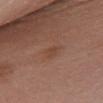Q: Was this lesion biopsied?
A: imaged on a skin check; not biopsied
Q: Lesion location?
A: the chest
Q: How large is the lesion?
A: ≈2.5 mm
Q: What is the imaging modality?
A: ~15 mm crop, total-body skin-cancer survey
Q: What did automated image analysis measure?
A: border irregularity of about 2 on a 0–10 scale and internal color variation of about 2 on a 0–10 scale; a nevus-likeness score of about 0/100
Q: What are the patient's age and sex?
A: female, approximately 50 years of age
Q: Illumination type?
A: white-light